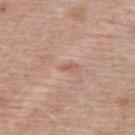Findings:
- workup · no biopsy performed (imaged during a skin exam)
- automated lesion analysis · an average lesion color of about L≈57 a*≈25 b*≈27 (CIELAB) and a normalized lesion–skin contrast near 6; lesion-presence confidence of about 100/100
- image source · 15 mm crop, total-body photography
- size · ~1.5 mm (longest diameter)
- tile lighting · white-light
- site · the upper back
- subject · female, about 50 years old